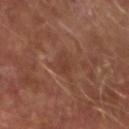workup = no biopsy performed (imaged during a skin exam); location = the right forearm; patient = male, about 65 years old; image source = 15 mm crop, total-body photography.